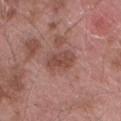TBP lesion metrics=a lesion area of about 7 mm², a shape eccentricity near 0.85, and a symmetry-axis asymmetry near 0.15; border irregularity of about 2 on a 0–10 scale, a within-lesion color-variation index near 2.5/10, and a peripheral color-asymmetry measure near 1 | subject=male, aged approximately 55 | image=~15 mm tile from a whole-body skin photo | tile lighting=white-light illumination | location=the lower back.